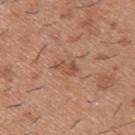Q: Is there a histopathology result?
A: total-body-photography surveillance lesion; no biopsy
Q: Illumination type?
A: white-light
Q: Where on the body is the lesion?
A: the back
Q: What is the imaging modality?
A: ~15 mm tile from a whole-body skin photo
Q: What did automated image analysis measure?
A: an average lesion color of about L≈51 a*≈23 b*≈31 (CIELAB), about 8 CIELAB-L* units darker than the surrounding skin, and a normalized lesion–skin contrast near 6; a nevus-likeness score of about 0/100 and lesion-presence confidence of about 100/100
Q: What are the patient's age and sex?
A: male, about 40 years old